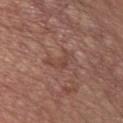Approximately 4 mm at its widest. On the chest. This is a white-light tile. A lesion tile, about 15 mm wide, cut from a 3D total-body photograph. The subject is a male about 55 years old.